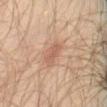Captured during whole-body skin photography for melanoma surveillance; the lesion was not biopsied.
Automated image analysis of the tile measured a lesion area of about 4 mm², an outline eccentricity of about 0.9 (0 = round, 1 = elongated), and two-axis asymmetry of about 0.2. It also reported a lesion–skin lightness drop of about 8 and a normalized lesion–skin contrast near 5.5.
This image is a 15 mm lesion crop taken from a total-body photograph.
The tile uses cross-polarized illumination.
The patient is a male about 30 years old.
The lesion is located on the left leg.
The recorded lesion diameter is about 3 mm.